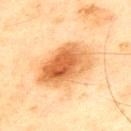notes — catalogued during a skin exam; not biopsied
imaging modality — ~15 mm tile from a whole-body skin photo
image-analysis metrics — an area of roughly 25 mm², an outline eccentricity of about 0.75 (0 = round, 1 = elongated), and a shape-asymmetry score of about 0.15 (0 = symmetric); a lesion color around L≈54 a*≈22 b*≈39 in CIELAB, about 13 CIELAB-L* units darker than the surrounding skin, and a normalized lesion–skin contrast near 9; border irregularity of about 2 on a 0–10 scale, a color-variation rating of about 8/10, and peripheral color asymmetry of about 2.5; an automated nevus-likeness rating near 100 out of 100
subject — male, in their mid-40s
site — the upper back
tile lighting — cross-polarized illumination